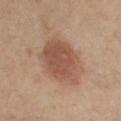Captured during whole-body skin photography for melanoma surveillance; the lesion was not biopsied. A female subject, aged around 50. The lesion is located on the left upper arm. Cropped from a whole-body photographic skin survey; the tile spans about 15 mm. Automated image analysis of the tile measured a footprint of about 19 mm², an outline eccentricity of about 0.7 (0 = round, 1 = elongated), and a symmetry-axis asymmetry near 0.15. The analysis additionally found an average lesion color of about L≈49 a*≈20 b*≈27 (CIELAB), a lesion–skin lightness drop of about 10, and a normalized border contrast of about 7.5. Imaged with cross-polarized lighting. Measured at roughly 6 mm in maximum diameter.